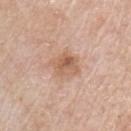biopsy_status: not biopsied; imaged during a skin examination
automated_metrics:
  area_mm2_approx: 7.0
  eccentricity: 0.55
  shape_asymmetry: 0.15
  cielab_L: 59
  cielab_a: 20
  cielab_b: 31
patient:
  sex: male
  age_approx: 65
image:
  source: total-body photography crop
  field_of_view_mm: 15
lesion_size:
  long_diameter_mm_approx: 3.0
site: chest